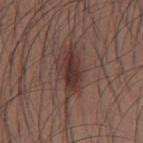This lesion was catalogued during total-body skin photography and was not selected for biopsy.
On the mid back.
The total-body-photography lesion software estimated a mean CIELAB color near L≈33 a*≈19 b*≈20, about 11 CIELAB-L* units darker than the surrounding skin, and a normalized lesion–skin contrast near 10. And it measured a classifier nevus-likeness of about 95/100 and lesion-presence confidence of about 100/100.
A male patient, aged approximately 35.
Approximately 4.5 mm at its widest.
Imaged with white-light lighting.
A 15 mm crop from a total-body photograph taken for skin-cancer surveillance.